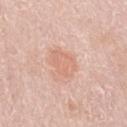workup — catalogued during a skin exam; not biopsied | image — 15 mm crop, total-body photography | location — the left upper arm | subject — female, about 65 years old.